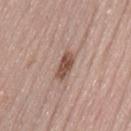<case>
  <biopsy_status>not biopsied; imaged during a skin examination</biopsy_status>
  <site>lower back</site>
  <image>
    <source>total-body photography crop</source>
    <field_of_view_mm>15</field_of_view_mm>
  </image>
  <patient>
    <sex>female</sex>
    <age_approx>50</age_approx>
  </patient>
  <lesion_size>
    <long_diameter_mm_approx>3.5</long_diameter_mm_approx>
  </lesion_size>
  <lighting>white-light</lighting>
  <automated_metrics>
    <area_mm2_approx>4.5</area_mm2_approx>
    <eccentricity>0.85</eccentricity>
    <shape_asymmetry>0.25</shape_asymmetry>
    <cielab_L>50</cielab_L>
    <cielab_a>20</cielab_a>
    <cielab_b>26</cielab_b>
    <vs_skin_darker_L>14.0</vs_skin_darker_L>
    <nevus_likeness_0_100>80</nevus_likeness_0_100>
    <lesion_detection_confidence_0_100>100</lesion_detection_confidence_0_100>
  </automated_metrics>
</case>